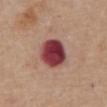{"automated_metrics": {"area_mm2_approx": 13.0, "border_irregularity_0_10": 1.0, "color_variation_0_10": 6.5, "peripheral_color_asymmetry": 2.5}, "patient": {"sex": "male", "age_approx": 65}, "lighting": "white-light", "lesion_size": {"long_diameter_mm_approx": 4.5}, "site": "mid back", "image": {"source": "total-body photography crop", "field_of_view_mm": 15}}